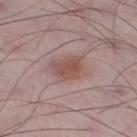Case summary:
– follow-up — imaged on a skin check; not biopsied
– patient — male, approximately 50 years of age
– image source — ~15 mm tile from a whole-body skin photo
– location — the right thigh